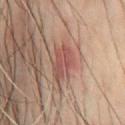Assessment: No biopsy was performed on this lesion — it was imaged during a full skin examination and was not determined to be concerning. Image and clinical context: About 4.5 mm across. An algorithmic analysis of the crop reported an average lesion color of about L≈45 a*≈21 b*≈23 (CIELAB). A roughly 15 mm field-of-view crop from a total-body skin photograph. The lesion is on the chest. A male patient approximately 60 years of age. This is a cross-polarized tile.